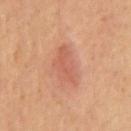Q: Was a biopsy performed?
A: no biopsy performed (imaged during a skin exam)
Q: What lighting was used for the tile?
A: cross-polarized
Q: What kind of image is this?
A: ~15 mm crop, total-body skin-cancer survey
Q: Lesion location?
A: the mid back
Q: Lesion size?
A: ~5 mm (longest diameter)
Q: Patient demographics?
A: male, aged 68–72